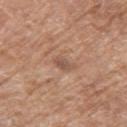Case summary:
• follow-up · imaged on a skin check; not biopsied
• acquisition · ~15 mm tile from a whole-body skin photo
• illumination · white-light
• lesion size · about 2.5 mm
• patient · female, in their mid-70s
• location · the leg
• TBP lesion metrics · a lesion area of about 3 mm² and an eccentricity of roughly 0.8; a lesion color around L≈53 a*≈19 b*≈28 in CIELAB and a normalized border contrast of about 6; border irregularity of about 3 on a 0–10 scale, a within-lesion color-variation index near 1.5/10, and peripheral color asymmetry of about 0.5; an automated nevus-likeness rating near 0 out of 100 and lesion-presence confidence of about 100/100